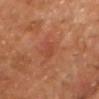biopsy status: imaged on a skin check; not biopsied
size: about 2.5 mm
lighting: cross-polarized illumination
acquisition: ~15 mm crop, total-body skin-cancer survey
TBP lesion metrics: a footprint of about 3 mm², an eccentricity of roughly 0.85, and a shape-asymmetry score of about 0.3 (0 = symmetric); a lesion color around L≈43 a*≈27 b*≈32 in CIELAB; border irregularity of about 2.5 on a 0–10 scale and a color-variation rating of about 1/10
subject: male, in their mid- to late 60s
body site: the chest